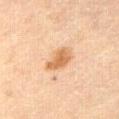Clinical summary: About 3.5 mm across. Located on the front of the torso. A female patient about 55 years old. A roughly 15 mm field-of-view crop from a total-body skin photograph. The tile uses cross-polarized illumination.